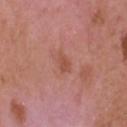Part of a total-body skin-imaging series; this lesion was reviewed on a skin check and was not flagged for biopsy.
Imaged with white-light lighting.
Automated tile analysis of the lesion measured a mean CIELAB color near L≈52 a*≈26 b*≈30, roughly 7 lightness units darker than nearby skin, and a normalized lesion–skin contrast near 6. The analysis additionally found a classifier nevus-likeness of about 0/100 and lesion-presence confidence of about 100/100.
On the head or neck.
A male subject, about 50 years old.
The recorded lesion diameter is about 2.5 mm.
A 15 mm crop from a total-body photograph taken for skin-cancer surveillance.